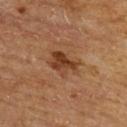workup: total-body-photography surveillance lesion; no biopsy
site: the back
illumination: cross-polarized illumination
imaging modality: ~15 mm tile from a whole-body skin photo
size: ~4 mm (longest diameter)
patient: male, in their mid- to late 60s